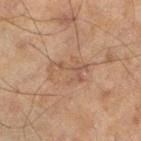{"patient": {"sex": "male", "age_approx": 45}, "image": {"source": "total-body photography crop", "field_of_view_mm": 15}, "lesion_size": {"long_diameter_mm_approx": 4.5}, "site": "leg", "lighting": "cross-polarized"}